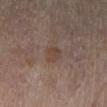Clinical impression: The lesion was tiled from a total-body skin photograph and was not biopsied. Clinical summary: A female patient in their mid-70s. A 15 mm close-up extracted from a 3D total-body photography capture. The tile uses cross-polarized illumination. The lesion is located on the right lower leg.